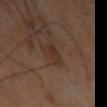Assessment:
No biopsy was performed on this lesion — it was imaged during a full skin examination and was not determined to be concerning.
Background:
An algorithmic analysis of the crop reported an area of roughly 4 mm² and a shape eccentricity near 0.7. The software also gave an average lesion color of about L≈30 a*≈16 b*≈24 (CIELAB) and about 5 CIELAB-L* units darker than the surrounding skin. The software also gave a border-irregularity index near 2/10 and internal color variation of about 2.5 on a 0–10 scale. It also reported an automated nevus-likeness rating near 5 out of 100 and a lesion-detection confidence of about 100/100. A male subject, aged 38 to 42. Cropped from a whole-body photographic skin survey; the tile spans about 15 mm. Captured under cross-polarized illumination. The lesion's longest dimension is about 3 mm. Located on the left upper arm.